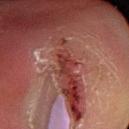patient: female, aged around 50 | site: the left lower leg | TBP lesion metrics: a border-irregularity index near 6/10 and a peripheral color-asymmetry measure near 0; a nevus-likeness score of about 0/100 and lesion-presence confidence of about 80/100 | imaging modality: ~15 mm tile from a whole-body skin photo.